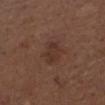No biopsy was performed on this lesion — it was imaged during a full skin examination and was not determined to be concerning. Automated image analysis of the tile measured a footprint of about 4.5 mm² and a symmetry-axis asymmetry near 0.3. A 15 mm close-up tile from a total-body photography series done for melanoma screening. From the head or neck. The lesion's longest dimension is about 3 mm. Imaged with white-light lighting. A male subject aged 73–77.